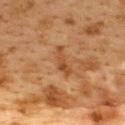Assessment: Captured during whole-body skin photography for melanoma surveillance; the lesion was not biopsied. Acquisition and patient details: The lesion is located on the back. Automated image analysis of the tile measured an area of roughly 3.5 mm² and an eccentricity of roughly 0.95. The software also gave a mean CIELAB color near L≈40 a*≈20 b*≈33, roughly 7 lightness units darker than nearby skin, and a normalized lesion–skin contrast near 6.5. It also reported a border-irregularity rating of about 5.5/10 and peripheral color asymmetry of about 0. And it measured a classifier nevus-likeness of about 0/100 and lesion-presence confidence of about 100/100. About 3.5 mm across. The tile uses cross-polarized illumination. A 15 mm close-up tile from a total-body photography series done for melanoma screening. A female subject, aged approximately 40.